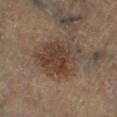• follow-up · imaged on a skin check; not biopsied
• image-analysis metrics · a color-variation rating of about 6/10 and peripheral color asymmetry of about 2; a nevus-likeness score of about 75/100 and lesion-presence confidence of about 100/100
• size · ≈6 mm
• imaging modality · total-body-photography crop, ~15 mm field of view
• illumination · cross-polarized illumination
• subject · male, aged 63 to 67
• body site · the right lower leg